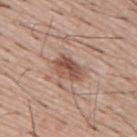notes = catalogued during a skin exam; not biopsied | image = ~15 mm crop, total-body skin-cancer survey | lighting = white-light | automated metrics = an automated nevus-likeness rating near 65 out of 100 and lesion-presence confidence of about 100/100 | subject = male, approximately 55 years of age.